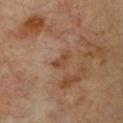This lesion was catalogued during total-body skin photography and was not selected for biopsy.
Captured under cross-polarized illumination.
Cropped from a total-body skin-imaging series; the visible field is about 15 mm.
An algorithmic analysis of the crop reported a nevus-likeness score of about 0/100 and lesion-presence confidence of about 100/100.
A female subject, about 55 years old.
Located on the chest.
The recorded lesion diameter is about 2.5 mm.